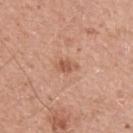Assessment: Part of a total-body skin-imaging series; this lesion was reviewed on a skin check and was not flagged for biopsy. Background: Imaged with white-light lighting. The patient is a male about 40 years old. The lesion is located on the left upper arm. A lesion tile, about 15 mm wide, cut from a 3D total-body photograph. The recorded lesion diameter is about 2.5 mm.